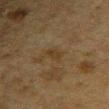Part of a total-body skin-imaging series; this lesion was reviewed on a skin check and was not flagged for biopsy.